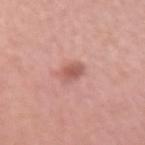{"patient": {"sex": "male", "age_approx": 60}, "site": "left forearm", "image": {"source": "total-body photography crop", "field_of_view_mm": 15}}